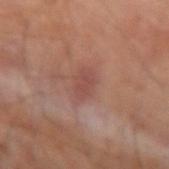Recorded during total-body skin imaging; not selected for excision or biopsy. The subject is a male aged 63 to 67. Imaged with cross-polarized lighting. The lesion is located on the left forearm. Cropped from a total-body skin-imaging series; the visible field is about 15 mm.